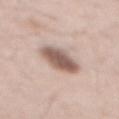The lesion was tiled from a total-body skin photograph and was not biopsied. Captured under white-light illumination. A 15 mm crop from a total-body photograph taken for skin-cancer surveillance. Located on the mid back. The subject is a male about 40 years old. The recorded lesion diameter is about 5.5 mm.